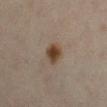<lesion>
<biopsy_status>not biopsied; imaged during a skin examination</biopsy_status>
<lighting>cross-polarized</lighting>
<automated_metrics>
  <cielab_L>35</cielab_L>
  <cielab_a>14</cielab_a>
  <cielab_b>24</cielab_b>
  <border_irregularity_0_10>1.5</border_irregularity_0_10>
  <color_variation_0_10>3.5</color_variation_0_10>
  <peripheral_color_asymmetry>1.0</peripheral_color_asymmetry>
  <nevus_likeness_0_100>100</nevus_likeness_0_100>
  <lesion_detection_confidence_0_100>100</lesion_detection_confidence_0_100>
</automated_metrics>
<patient>
  <sex>female</sex>
  <age_approx>45</age_approx>
</patient>
<site>right lower leg</site>
<lesion_size>
  <long_diameter_mm_approx>2.5</long_diameter_mm_approx>
</lesion_size>
<image>
  <source>total-body photography crop</source>
  <field_of_view_mm>15</field_of_view_mm>
</image>
</lesion>